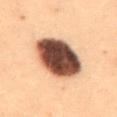Findings:
• workup: imaged on a skin check; not biopsied
• image source: total-body-photography crop, ~15 mm field of view
• diameter: ~5.5 mm (longest diameter)
• subject: female, about 20 years old
• anatomic site: the abdomen
• automated lesion analysis: a mean CIELAB color near L≈36 a*≈17 b*≈23, about 25 CIELAB-L* units darker than the surrounding skin, and a normalized border contrast of about 18.5; a border-irregularity index near 1/10, a color-variation rating of about 7.5/10, and peripheral color asymmetry of about 2